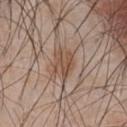Captured during whole-body skin photography for melanoma surveillance; the lesion was not biopsied. Captured under white-light illumination. A region of skin cropped from a whole-body photographic capture, roughly 15 mm wide. Automated image analysis of the tile measured an eccentricity of roughly 0.65. And it measured a lesion color around L≈50 a*≈17 b*≈28 in CIELAB, a lesion–skin lightness drop of about 9, and a normalized border contrast of about 7. And it measured a border-irregularity index near 5/10 and a color-variation rating of about 2.5/10. It also reported a classifier nevus-likeness of about 50/100. On the chest. A male patient, about 45 years old. Approximately 3.5 mm at its widest.